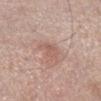Clinical impression:
Imaged during a routine full-body skin examination; the lesion was not biopsied and no histopathology is available.
Context:
A close-up tile cropped from a whole-body skin photograph, about 15 mm across. The total-body-photography lesion software estimated a lesion area of about 5 mm² and a symmetry-axis asymmetry near 0.3. The software also gave a border-irregularity rating of about 3.5/10. The patient is a male approximately 55 years of age. The lesion is on the right lower leg. About 3 mm across.